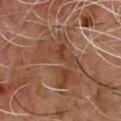Impression: This lesion was catalogued during total-body skin photography and was not selected for biopsy. Acquisition and patient details: Imaged with cross-polarized lighting. Cropped from a total-body skin-imaging series; the visible field is about 15 mm. The subject is a male roughly 65 years of age. Automated image analysis of the tile measured a mean CIELAB color near L≈41 a*≈20 b*≈29, about 6 CIELAB-L* units darker than the surrounding skin, and a lesion-to-skin contrast of about 5.5 (normalized; higher = more distinct). It also reported a detector confidence of about 80 out of 100 that the crop contains a lesion. On the chest.